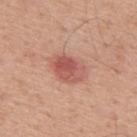<tbp_lesion>
  <biopsy_status>not biopsied; imaged during a skin examination</biopsy_status>
  <patient>
    <sex>male</sex>
    <age_approx>55</age_approx>
  </patient>
  <image>
    <source>total-body photography crop</source>
    <field_of_view_mm>15</field_of_view_mm>
  </image>
  <lighting>white-light</lighting>
  <site>back</site>
  <automated_metrics>
    <cielab_L>55</cielab_L>
    <cielab_a>28</cielab_a>
    <cielab_b>27</cielab_b>
    <vs_skin_darker_L>11.0</vs_skin_darker_L>
    <vs_skin_contrast_norm>7.5</vs_skin_contrast_norm>
    <border_irregularity_0_10>1.5</border_irregularity_0_10>
    <color_variation_0_10>6.0</color_variation_0_10>
    <peripheral_color_asymmetry>2.0</peripheral_color_asymmetry>
    <nevus_likeness_0_100>75</nevus_likeness_0_100>
  </automated_metrics>
  <lesion_size>
    <long_diameter_mm_approx>4.0</long_diameter_mm_approx>
  </lesion_size>
</tbp_lesion>